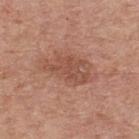Clinical impression: No biopsy was performed on this lesion — it was imaged during a full skin examination and was not determined to be concerning. Acquisition and patient details: A male subject approximately 80 years of age. About 5.5 mm across. From the upper back. A 15 mm close-up tile from a total-body photography series done for melanoma screening. An algorithmic analysis of the crop reported a footprint of about 12 mm², a shape eccentricity near 0.85, and two-axis asymmetry of about 0.35. The software also gave roughly 8 lightness units darker than nearby skin and a normalized border contrast of about 6. And it measured a border-irregularity rating of about 4/10, internal color variation of about 3 on a 0–10 scale, and radial color variation of about 1.